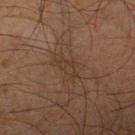The patient is a male aged 63 to 67. Approximately 3.5 mm at its widest. Automated image analysis of the tile measured about 4 CIELAB-L* units darker than the surrounding skin and a normalized border contrast of about 5. A region of skin cropped from a whole-body photographic capture, roughly 15 mm wide. The lesion is located on the leg. Captured under cross-polarized illumination.